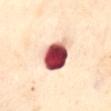{
  "biopsy_status": "not biopsied; imaged during a skin examination"
}